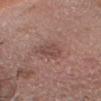Clinical impression: The lesion was photographed on a routine skin check and not biopsied; there is no pathology result. Background: The lesion is on the head or neck. The total-body-photography lesion software estimated a symmetry-axis asymmetry near 0.25. The software also gave an average lesion color of about L≈47 a*≈20 b*≈22 (CIELAB) and a normalized lesion–skin contrast near 5.5. The software also gave a within-lesion color-variation index near 2.5/10 and a peripheral color-asymmetry measure near 1. The patient is a male about 55 years old. A lesion tile, about 15 mm wide, cut from a 3D total-body photograph. Longest diameter approximately 3.5 mm.